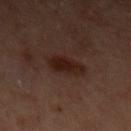{
  "site": "arm",
  "patient": {
    "sex": "female",
    "age_approx": 45
  },
  "image": {
    "source": "total-body photography crop",
    "field_of_view_mm": 15
  },
  "lighting": "cross-polarized",
  "lesion_size": {
    "long_diameter_mm_approx": 4.0
  }
}